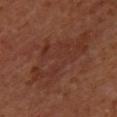Q: Was a biopsy performed?
A: imaged on a skin check; not biopsied
Q: What are the patient's age and sex?
A: female, aged 38 to 42
Q: How was this image acquired?
A: 15 mm crop, total-body photography
Q: What is the lesion's diameter?
A: about 10 mm
Q: How was the tile lit?
A: cross-polarized illumination
Q: What is the anatomic site?
A: the back